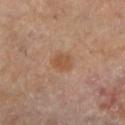  biopsy_status: not biopsied; imaged during a skin examination
  lighting: cross-polarized
  patient:
    sex: female
    age_approx: 70
  image:
    source: total-body photography crop
    field_of_view_mm: 15
  lesion_size:
    long_diameter_mm_approx: 2.5
  automated_metrics:
    area_mm2_approx: 4.0
    eccentricity: 0.7
    nevus_likeness_0_100: 65
  site: left lower leg